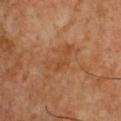This lesion was catalogued during total-body skin photography and was not selected for biopsy.
A 15 mm crop from a total-body photograph taken for skin-cancer surveillance.
The lesion's longest dimension is about 4 mm.
A male subject, in their 50s.
Captured under cross-polarized illumination.
The lesion is on the chest.
The lesion-visualizer software estimated a detector confidence of about 100 out of 100 that the crop contains a lesion.